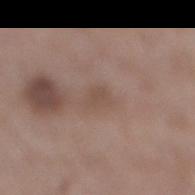Clinical impression: This lesion was catalogued during total-body skin photography and was not selected for biopsy. Acquisition and patient details: About 2.5 mm across. A lesion tile, about 15 mm wide, cut from a 3D total-body photograph. A male subject, aged approximately 50. This is a white-light tile. Located on the right lower leg.